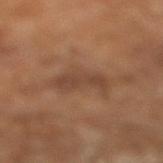Recorded during total-body skin imaging; not selected for excision or biopsy. A male subject aged around 65. The tile uses cross-polarized illumination. A close-up tile cropped from a whole-body skin photograph, about 15 mm across.